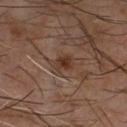Recorded during total-body skin imaging; not selected for excision or biopsy. Captured under cross-polarized illumination. The subject is a male aged 58–62. The recorded lesion diameter is about 3 mm. Located on the chest. A 15 mm crop from a total-body photograph taken for skin-cancer surveillance.